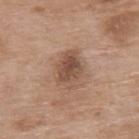This lesion was catalogued during total-body skin photography and was not selected for biopsy.
Automated image analysis of the tile measured an area of roughly 9.5 mm², an outline eccentricity of about 0.75 (0 = round, 1 = elongated), and two-axis asymmetry of about 0.15. It also reported a border-irregularity rating of about 1.5/10, a color-variation rating of about 5/10, and radial color variation of about 2. And it measured an automated nevus-likeness rating near 40 out of 100 and lesion-presence confidence of about 100/100.
A lesion tile, about 15 mm wide, cut from a 3D total-body photograph.
Imaged with white-light lighting.
Located on the back.
The lesion's longest dimension is about 4 mm.
A female patient about 75 years old.